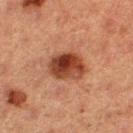Image and clinical context: A female subject aged 38 to 42. The lesion-visualizer software estimated a color-variation rating of about 8/10 and radial color variation of about 3. The lesion is on the right thigh. This is a cross-polarized tile. A roughly 15 mm field-of-view crop from a total-body skin photograph. The recorded lesion diameter is about 4 mm.